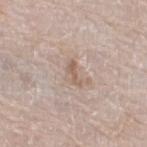biopsy status = imaged on a skin check; not biopsied | body site = the left lower leg | lighting = white-light illumination | diameter = ≈2.5 mm | patient = male, aged 78 to 82 | image source = ~15 mm tile from a whole-body skin photo.